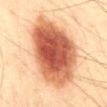No biopsy was performed on this lesion — it was imaged during a full skin examination and was not determined to be concerning.
Approximately 10.5 mm at its widest.
The lesion-visualizer software estimated a lesion color around L≈55 a*≈25 b*≈34 in CIELAB and a lesion-to-skin contrast of about 12.5 (normalized; higher = more distinct). And it measured border irregularity of about 2 on a 0–10 scale, a color-variation rating of about 7.5/10, and peripheral color asymmetry of about 2.
The subject is a male aged approximately 40.
On the front of the torso.
Cropped from a whole-body photographic skin survey; the tile spans about 15 mm.